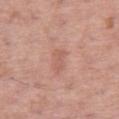Q: Is there a histopathology result?
A: catalogued during a skin exam; not biopsied
Q: Where on the body is the lesion?
A: the right thigh
Q: What is the lesion's diameter?
A: ≈3 mm
Q: What kind of image is this?
A: total-body-photography crop, ~15 mm field of view
Q: What did automated image analysis measure?
A: an area of roughly 3.5 mm² and a shape-asymmetry score of about 0.3 (0 = symmetric); a color-variation rating of about 1.5/10 and peripheral color asymmetry of about 0
Q: Illumination type?
A: white-light illumination
Q: Patient demographics?
A: female, aged 58 to 62